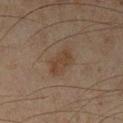Context:
About 4 mm across. The patient is a male in their mid- to late 40s. A close-up tile cropped from a whole-body skin photograph, about 15 mm across. Captured under cross-polarized illumination. The lesion is located on the left lower leg.